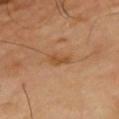biopsy status — imaged on a skin check; not biopsied.